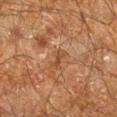Assessment:
Captured during whole-body skin photography for melanoma surveillance; the lesion was not biopsied.
Context:
The tile uses cross-polarized illumination. A male patient, approximately 60 years of age. On the right lower leg. A 15 mm close-up extracted from a 3D total-body photography capture. Longest diameter approximately 2.5 mm.